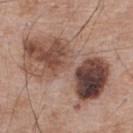Assessment: Captured during whole-body skin photography for melanoma surveillance; the lesion was not biopsied. Context: The tile uses white-light illumination. The patient is a male roughly 65 years of age. A close-up tile cropped from a whole-body skin photograph, about 15 mm across. On the upper back.